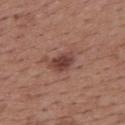- notes — catalogued during a skin exam; not biopsied
- TBP lesion metrics — a mean CIELAB color near L≈42 a*≈22 b*≈24 and a lesion–skin lightness drop of about 11; an automated nevus-likeness rating near 80 out of 100 and lesion-presence confidence of about 100/100
- diameter — about 3.5 mm
- image source — 15 mm crop, total-body photography
- body site — the back
- subject — female, aged around 50
- illumination — white-light illumination